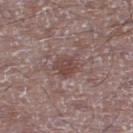Notes:
- biopsy status · imaged on a skin check; not biopsied
- location · the leg
- image-analysis metrics · a footprint of about 6 mm² and a shape eccentricity near 0.4; a border-irregularity index near 2.5/10 and a within-lesion color-variation index near 2.5/10; a nevus-likeness score of about 15/100 and lesion-presence confidence of about 100/100
- tile lighting · white-light illumination
- acquisition · ~15 mm crop, total-body skin-cancer survey
- patient · male, in their 50s